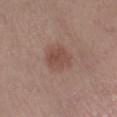biopsy status=imaged on a skin check; not biopsied
patient=female, aged around 40
acquisition=15 mm crop, total-body photography
location=the left lower leg
lighting=white-light illumination
size=≈3 mm